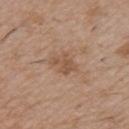workup: imaged on a skin check; not biopsied
location: the chest
acquisition: ~15 mm crop, total-body skin-cancer survey
patient: male, aged around 50